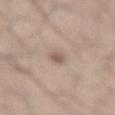- patient — male, aged 23–27
- site — the lower back
- lesion diameter — ~2.5 mm (longest diameter)
- lighting — white-light
- image source — ~15 mm crop, total-body skin-cancer survey